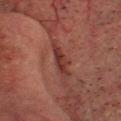Part of a total-body skin-imaging series; this lesion was reviewed on a skin check and was not flagged for biopsy.
The lesion is located on the head or neck.
A close-up tile cropped from a whole-body skin photograph, about 15 mm across.
A male subject aged 58–62.
Automated tile analysis of the lesion measured border irregularity of about 3.5 on a 0–10 scale, internal color variation of about 1.5 on a 0–10 scale, and a peripheral color-asymmetry measure near 0.5.
The lesion's longest dimension is about 4 mm.